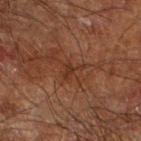Clinical impression: No biopsy was performed on this lesion — it was imaged during a full skin examination and was not determined to be concerning. Background: The subject is a male approximately 65 years of age. On the right forearm. Cropped from a total-body skin-imaging series; the visible field is about 15 mm.